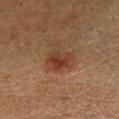{"biopsy_status": "not biopsied; imaged during a skin examination", "patient": {"sex": "female", "age_approx": 50}, "lighting": "cross-polarized", "site": "right lower leg", "lesion_size": {"long_diameter_mm_approx": 3.5}, "image": {"source": "total-body photography crop", "field_of_view_mm": 15}}